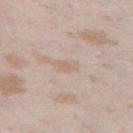Q: Was a biopsy performed?
A: catalogued during a skin exam; not biopsied
Q: Lesion size?
A: ~2.5 mm (longest diameter)
Q: What is the anatomic site?
A: the left thigh
Q: What lighting was used for the tile?
A: white-light
Q: Who is the patient?
A: female, in their mid- to late 20s
Q: Automated lesion metrics?
A: a classifier nevus-likeness of about 0/100 and lesion-presence confidence of about 95/100
Q: How was this image acquired?
A: 15 mm crop, total-body photography